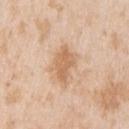{"biopsy_status": "not biopsied; imaged during a skin examination", "image": {"source": "total-body photography crop", "field_of_view_mm": 15}, "automated_metrics": {"area_mm2_approx": 8.5, "eccentricity": 0.75, "cielab_L": 65, "cielab_a": 19, "cielab_b": 35, "vs_skin_darker_L": 10.0, "nevus_likeness_0_100": 15, "lesion_detection_confidence_0_100": 100}, "site": "left upper arm", "lighting": "white-light", "lesion_size": {"long_diameter_mm_approx": 4.5}, "patient": {"sex": "male", "age_approx": 45}}